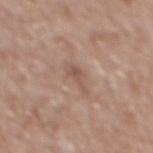Findings:
* biopsy status · total-body-photography surveillance lesion; no biopsy
* site · the back
* subject · male, aged around 65
* acquisition · ~15 mm tile from a whole-body skin photo